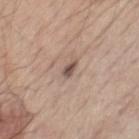An algorithmic analysis of the crop reported an average lesion color of about L≈51 a*≈16 b*≈22 (CIELAB) and about 13 CIELAB-L* units darker than the surrounding skin. The software also gave an automated nevus-likeness rating near 20 out of 100 and a lesion-detection confidence of about 100/100. A male patient aged 58 to 62. The lesion is on the chest. About 2.5 mm across. A roughly 15 mm field-of-view crop from a total-body skin photograph. Captured under white-light illumination.